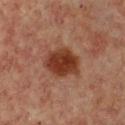Impression: The lesion was photographed on a routine skin check and not biopsied; there is no pathology result. Acquisition and patient details: A close-up tile cropped from a whole-body skin photograph, about 15 mm across. A female subject, aged 43–47. Imaged with cross-polarized lighting. Located on the chest. About 4.5 mm across.